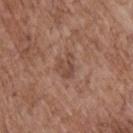Assessment:
Imaged during a routine full-body skin examination; the lesion was not biopsied and no histopathology is available.
Clinical summary:
The tile uses white-light illumination. The lesion is on the upper back. Approximately 3 mm at its widest. A male patient, aged approximately 70. Cropped from a whole-body photographic skin survey; the tile spans about 15 mm.